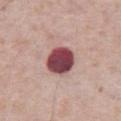Clinical impression:
The lesion was tiled from a total-body skin photograph and was not biopsied.
Image and clinical context:
The subject is a male approximately 75 years of age. A 15 mm close-up extracted from a 3D total-body photography capture. On the front of the torso.